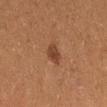workup: total-body-photography surveillance lesion; no biopsy | lesion diameter: ~2.5 mm (longest diameter) | location: the right thigh | automated lesion analysis: a footprint of about 4.5 mm², an outline eccentricity of about 0.65 (0 = round, 1 = elongated), and a shape-asymmetry score of about 0.2 (0 = symmetric); a lesion-to-skin contrast of about 7.5 (normalized; higher = more distinct); a classifier nevus-likeness of about 95/100 and a detector confidence of about 100 out of 100 that the crop contains a lesion | image source: total-body-photography crop, ~15 mm field of view | subject: female, approximately 50 years of age.